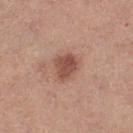The lesion was tiled from a total-body skin photograph and was not biopsied.
From the left thigh.
Approximately 3 mm at its widest.
The subject is a female about 40 years old.
The tile uses white-light illumination.
A 15 mm close-up extracted from a 3D total-body photography capture.